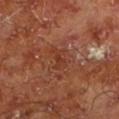Findings:
– workup · imaged on a skin check; not biopsied
– image source · ~15 mm tile from a whole-body skin photo
– diameter · ~3 mm (longest diameter)
– automated lesion analysis · border irregularity of about 5.5 on a 0–10 scale, a within-lesion color-variation index near 0.5/10, and peripheral color asymmetry of about 0; an automated nevus-likeness rating near 0 out of 100 and a lesion-detection confidence of about 95/100
– site · the left lower leg
– subject · male, roughly 65 years of age